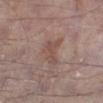{"biopsy_status": "not biopsied; imaged during a skin examination", "patient": {"sex": "male", "age_approx": 65}, "site": "right lower leg", "lighting": "white-light", "lesion_size": {"long_diameter_mm_approx": 3.5}, "image": {"source": "total-body photography crop", "field_of_view_mm": 15}}